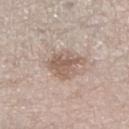Recorded during total-body skin imaging; not selected for excision or biopsy. A close-up tile cropped from a whole-body skin photograph, about 15 mm across. On the right lower leg. A male patient, roughly 70 years of age.